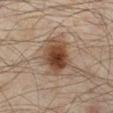Q: What is the lesion's diameter?
A: about 5 mm
Q: Who is the patient?
A: male, in their mid-40s
Q: What kind of image is this?
A: total-body-photography crop, ~15 mm field of view
Q: What is the anatomic site?
A: the left lower leg
Q: How was the tile lit?
A: cross-polarized